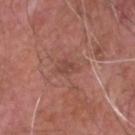Notes:
* follow-up · no biopsy performed (imaged during a skin exam)
* body site · the head or neck
* image-analysis metrics · an area of roughly 3.5 mm², a shape eccentricity near 0.85, and two-axis asymmetry of about 0.3
* imaging modality · ~15 mm tile from a whole-body skin photo
* diameter · ~2.5 mm (longest diameter)
* patient · male, aged around 75